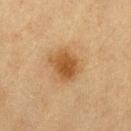  biopsy_status: not biopsied; imaged during a skin examination
  lesion_size:
    long_diameter_mm_approx: 4.0
  patient:
    sex: female
    age_approx: 40
  image:
    source: total-body photography crop
    field_of_view_mm: 15
  site: left thigh
  lighting: cross-polarized
  automated_metrics:
    cielab_L: 46
    cielab_a: 19
    cielab_b: 36
    vs_skin_darker_L: 10.0
    vs_skin_contrast_norm: 8.5